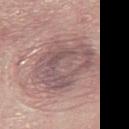notes: total-body-photography surveillance lesion; no biopsy | tile lighting: white-light illumination | patient: female, aged 63–67 | site: the chest | imaging modality: 15 mm crop, total-body photography.